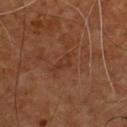Captured during whole-body skin photography for melanoma surveillance; the lesion was not biopsied.
A 15 mm close-up tile from a total-body photography series done for melanoma screening.
Longest diameter approximately 3 mm.
On the chest.
A male patient aged 53–57.